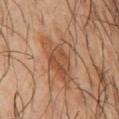{"image": {"source": "total-body photography crop", "field_of_view_mm": 15}, "lighting": "cross-polarized", "site": "chest", "patient": {"sex": "male", "age_approx": 60}}